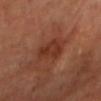{"biopsy_status": "not biopsied; imaged during a skin examination", "image": {"source": "total-body photography crop", "field_of_view_mm": 15}, "lesion_size": {"long_diameter_mm_approx": 4.5}, "lighting": "cross-polarized", "automated_metrics": {"border_irregularity_0_10": 3.0, "color_variation_0_10": 2.5}, "site": "head or neck"}